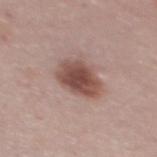Findings:
– biopsy status: catalogued during a skin exam; not biopsied
– subject: male, approximately 45 years of age
– location: the mid back
– image source: ~15 mm crop, total-body skin-cancer survey